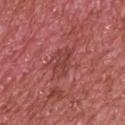Q: Is there a histopathology result?
A: total-body-photography surveillance lesion; no biopsy
Q: Illumination type?
A: white-light
Q: Lesion size?
A: ~4 mm (longest diameter)
Q: Automated lesion metrics?
A: an area of roughly 6 mm², an eccentricity of roughly 0.85, and two-axis asymmetry of about 0.3; about 7 CIELAB-L* units darker than the surrounding skin
Q: What are the patient's age and sex?
A: male, approximately 70 years of age
Q: Where on the body is the lesion?
A: the upper back
Q: What is the imaging modality?
A: total-body-photography crop, ~15 mm field of view